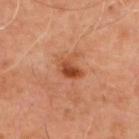No biopsy was performed on this lesion — it was imaged during a full skin examination and was not determined to be concerning.
A 15 mm close-up tile from a total-body photography series done for melanoma screening.
The lesion is located on the upper back.
A male patient aged 48–52.